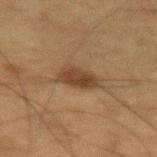  biopsy_status: not biopsied; imaged during a skin examination
  patient:
    sex: male
    age_approx: 75
  site: left thigh
  lighting: cross-polarized
  image:
    source: total-body photography crop
    field_of_view_mm: 15